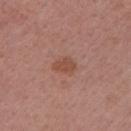Part of a total-body skin-imaging series; this lesion was reviewed on a skin check and was not flagged for biopsy. Measured at roughly 3 mm in maximum diameter. A female patient approximately 50 years of age. A region of skin cropped from a whole-body photographic capture, roughly 15 mm wide. This is a white-light tile. Located on the left upper arm.